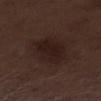Assessment: The lesion was photographed on a routine skin check and not biopsied; there is no pathology result. Image and clinical context: Longest diameter approximately 5.5 mm. The patient is a male about 70 years old. A region of skin cropped from a whole-body photographic capture, roughly 15 mm wide. The lesion is located on the right lower leg. Captured under white-light illumination.